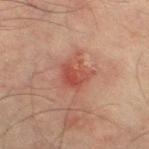Imaged during a routine full-body skin examination; the lesion was not biopsied and no histopathology is available. The lesion is on the right thigh. The subject is a male roughly 75 years of age. A region of skin cropped from a whole-body photographic capture, roughly 15 mm wide. The tile uses cross-polarized illumination. The recorded lesion diameter is about 3.5 mm. An algorithmic analysis of the crop reported a footprint of about 7.5 mm², a shape eccentricity near 0.4, and a symmetry-axis asymmetry near 0.35. The analysis additionally found about 8 CIELAB-L* units darker than the surrounding skin and a normalized border contrast of about 7. The software also gave a nevus-likeness score of about 0/100 and a lesion-detection confidence of about 100/100.